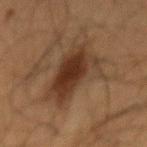Part of a total-body skin-imaging series; this lesion was reviewed on a skin check and was not flagged for biopsy. A male patient aged 63 to 67. The lesion is located on the mid back. Longest diameter approximately 8 mm. This is a cross-polarized tile. A 15 mm close-up tile from a total-body photography series done for melanoma screening. Automated image analysis of the tile measured an area of roughly 24 mm², an eccentricity of roughly 0.85, and a symmetry-axis asymmetry near 0.4. The software also gave a lesion color around L≈29 a*≈15 b*≈24 in CIELAB, a lesion–skin lightness drop of about 9, and a normalized lesion–skin contrast near 9. It also reported border irregularity of about 6 on a 0–10 scale, a within-lesion color-variation index near 5.5/10, and a peripheral color-asymmetry measure near 1.5.